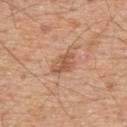Findings:
– workup · imaged on a skin check; not biopsied
– subject · male, in their mid- to late 50s
– lesion size · about 3 mm
– location · the upper back
– image · ~15 mm tile from a whole-body skin photo
– tile lighting · white-light illumination